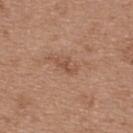This lesion was catalogued during total-body skin photography and was not selected for biopsy. Longest diameter approximately 3 mm. On the back. The patient is a female aged 38–42. This image is a 15 mm lesion crop taken from a total-body photograph. The lesion-visualizer software estimated a lesion area of about 3 mm², a shape eccentricity near 0.85, and a shape-asymmetry score of about 0.45 (0 = symmetric). It also reported an average lesion color of about L≈51 a*≈21 b*≈30 (CIELAB) and a lesion-to-skin contrast of about 5.5 (normalized; higher = more distinct). The analysis additionally found a border-irregularity index near 5.5/10, a color-variation rating of about 0/10, and radial color variation of about 0. The analysis additionally found a classifier nevus-likeness of about 0/100 and a lesion-detection confidence of about 100/100. Captured under white-light illumination.